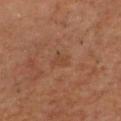workup: imaged on a skin check; not biopsied.